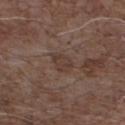Imaged during a routine full-body skin examination; the lesion was not biopsied and no histopathology is available. A region of skin cropped from a whole-body photographic capture, roughly 15 mm wide. The lesion is located on the chest. The patient is a male aged around 70. The lesion's longest dimension is about 2.5 mm. Imaged with white-light lighting.